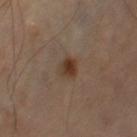- workup — total-body-photography surveillance lesion; no biopsy
- body site — the right thigh
- illumination — cross-polarized illumination
- image — ~15 mm tile from a whole-body skin photo
- lesion size — ≈2.5 mm